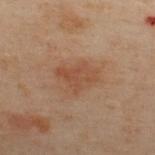Clinical impression:
This lesion was catalogued during total-body skin photography and was not selected for biopsy.
Clinical summary:
A male subject, aged 48–52. The lesion's longest dimension is about 4 mm. From the back. A roughly 15 mm field-of-view crop from a total-body skin photograph.